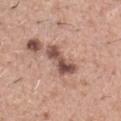Assessment: Recorded during total-body skin imaging; not selected for excision or biopsy. Acquisition and patient details: A 15 mm close-up extracted from a 3D total-body photography capture. From the chest. A male patient in their mid- to late 40s.